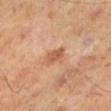Clinical impression:
Recorded during total-body skin imaging; not selected for excision or biopsy.
Clinical summary:
A male subject roughly 45 years of age. A region of skin cropped from a whole-body photographic capture, roughly 15 mm wide. On the left lower leg.